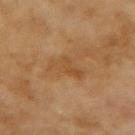| feature | finding |
|---|---|
| workup | no biopsy performed (imaged during a skin exam) |
| patient | female, aged around 60 |
| anatomic site | the arm |
| image | 15 mm crop, total-body photography |